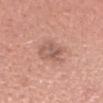Clinical impression: The lesion was photographed on a routine skin check and not biopsied; there is no pathology result. Clinical summary: Automated image analysis of the tile measured a footprint of about 11 mm². The software also gave a lesion color around L≈58 a*≈22 b*≈26 in CIELAB, about 9 CIELAB-L* units darker than the surrounding skin, and a normalized border contrast of about 6. The software also gave a border-irregularity rating of about 3/10, internal color variation of about 4.5 on a 0–10 scale, and peripheral color asymmetry of about 1.5. And it measured an automated nevus-likeness rating near 0 out of 100 and a lesion-detection confidence of about 100/100. About 4 mm across. A female patient, aged around 30. A lesion tile, about 15 mm wide, cut from a 3D total-body photograph. The lesion is on the head or neck.